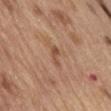Part of a total-body skin-imaging series; this lesion was reviewed on a skin check and was not flagged for biopsy.
A region of skin cropped from a whole-body photographic capture, roughly 15 mm wide.
A male patient aged 68–72.
The lesion is located on the abdomen.
The lesion-visualizer software estimated a mean CIELAB color near L≈51 a*≈20 b*≈32, a lesion–skin lightness drop of about 8, and a lesion-to-skin contrast of about 6.5 (normalized; higher = more distinct). The software also gave border irregularity of about 4.5 on a 0–10 scale and internal color variation of about 0 on a 0–10 scale. And it measured an automated nevus-likeness rating near 0 out of 100 and a detector confidence of about 100 out of 100 that the crop contains a lesion.
Imaged with white-light lighting.